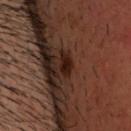Clinical impression: The lesion was photographed on a routine skin check and not biopsied; there is no pathology result. Background: A close-up tile cropped from a whole-body skin photograph, about 15 mm across. The lesion is located on the head or neck. Automated tile analysis of the lesion measured an average lesion color of about L≈19 a*≈19 b*≈22 (CIELAB) and a lesion–skin lightness drop of about 9. The software also gave a border-irregularity index near 2.5/10, internal color variation of about 3 on a 0–10 scale, and radial color variation of about 1. And it measured a nevus-likeness score of about 85/100 and lesion-presence confidence of about 100/100. The lesion's longest dimension is about 2.5 mm. Captured under cross-polarized illumination. The patient is a male about 45 years old.